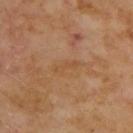This lesion was catalogued during total-body skin photography and was not selected for biopsy. Imaged with cross-polarized lighting. The lesion is on the upper back. A male patient aged 68–72. Cropped from a total-body skin-imaging series; the visible field is about 15 mm. The total-body-photography lesion software estimated about 5 CIELAB-L* units darker than the surrounding skin and a lesion-to-skin contrast of about 4.5 (normalized; higher = more distinct). The software also gave border irregularity of about 4.5 on a 0–10 scale, a within-lesion color-variation index near 0/10, and radial color variation of about 0. It also reported an automated nevus-likeness rating near 0 out of 100 and a lesion-detection confidence of about 100/100.